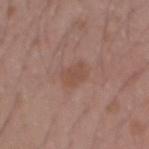Assessment: The lesion was photographed on a routine skin check and not biopsied; there is no pathology result. Background: Captured under white-light illumination. Cropped from a whole-body photographic skin survey; the tile spans about 15 mm. The lesion is located on the right forearm. The subject is a male aged approximately 20. An algorithmic analysis of the crop reported an area of roughly 5 mm², an eccentricity of roughly 0.65, and two-axis asymmetry of about 0.2. It also reported an average lesion color of about L≈49 a*≈20 b*≈26 (CIELAB), a lesion–skin lightness drop of about 6, and a lesion-to-skin contrast of about 5.5 (normalized; higher = more distinct). Measured at roughly 2.5 mm in maximum diameter.